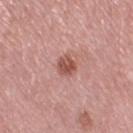The lesion's longest dimension is about 2.5 mm. A close-up tile cropped from a whole-body skin photograph, about 15 mm across. Imaged with white-light lighting. From the leg. A female patient aged 68 to 72. Automated image analysis of the tile measured an area of roughly 4.5 mm² and two-axis asymmetry of about 0.15. The software also gave a lesion–skin lightness drop of about 12 and a lesion-to-skin contrast of about 8.5 (normalized; higher = more distinct). The software also gave a border-irregularity rating of about 1.5/10, a within-lesion color-variation index near 3/10, and a peripheral color-asymmetry measure near 1.